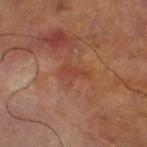<case>
  <biopsy_status>not biopsied; imaged during a skin examination</biopsy_status>
  <lighting>cross-polarized</lighting>
  <patient>
    <sex>male</sex>
    <age_approx>70</age_approx>
  </patient>
  <image>
    <source>total-body photography crop</source>
    <field_of_view_mm>15</field_of_view_mm>
  </image>
  <site>left lower leg</site>
</case>